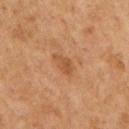Assessment: No biopsy was performed on this lesion — it was imaged during a full skin examination and was not determined to be concerning. Background: A 15 mm close-up tile from a total-body photography series done for melanoma screening. Imaged with cross-polarized lighting. A female subject, aged 58 to 62. The lesion is on the arm. Longest diameter approximately 3.5 mm. Automated tile analysis of the lesion measured an outline eccentricity of about 0.9 (0 = round, 1 = elongated) and a shape-asymmetry score of about 0.3 (0 = symmetric). And it measured a lesion color around L≈46 a*≈21 b*≈33 in CIELAB, about 7 CIELAB-L* units darker than the surrounding skin, and a lesion-to-skin contrast of about 6 (normalized; higher = more distinct). It also reported an automated nevus-likeness rating near 20 out of 100 and lesion-presence confidence of about 100/100.